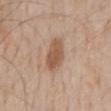| feature | finding |
|---|---|
| workup | imaged on a skin check; not biopsied |
| tile lighting | white-light |
| location | the back |
| subject | male, about 60 years old |
| image source | ~15 mm tile from a whole-body skin photo |